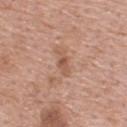Impression: Captured during whole-body skin photography for melanoma surveillance; the lesion was not biopsied. Clinical summary: The subject is a male about 55 years old. Automated tile analysis of the lesion measured a footprint of about 3 mm², an outline eccentricity of about 0.9 (0 = round, 1 = elongated), and a symmetry-axis asymmetry near 0.35. And it measured a mean CIELAB color near L≈54 a*≈22 b*≈30, roughly 9 lightness units darker than nearby skin, and a lesion-to-skin contrast of about 6.5 (normalized; higher = more distinct). Located on the upper back. A lesion tile, about 15 mm wide, cut from a 3D total-body photograph. Approximately 2.5 mm at its widest. Captured under white-light illumination.